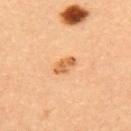<record>
<biopsy_status>not biopsied; imaged during a skin examination</biopsy_status>
<patient>
  <sex>female</sex>
  <age_approx>35</age_approx>
</patient>
<lesion_size>
  <long_diameter_mm_approx>3.0</long_diameter_mm_approx>
</lesion_size>
<site>upper back</site>
<image>
  <source>total-body photography crop</source>
  <field_of_view_mm>15</field_of_view_mm>
</image>
<lighting>cross-polarized</lighting>
</record>